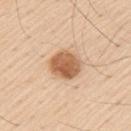| feature | finding |
|---|---|
| follow-up | catalogued during a skin exam; not biopsied |
| site | the arm |
| lighting | white-light |
| subject | male, approximately 60 years of age |
| automated lesion analysis | a nevus-likeness score of about 100/100 and a lesion-detection confidence of about 100/100 |
| imaging modality | total-body-photography crop, ~15 mm field of view |
| lesion size | ≈4 mm |